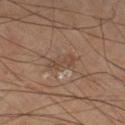Clinical impression:
Captured during whole-body skin photography for melanoma surveillance; the lesion was not biopsied.